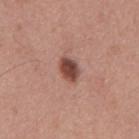workup: catalogued during a skin exam; not biopsied | image: ~15 mm tile from a whole-body skin photo | lesion diameter: ~3 mm (longest diameter) | illumination: white-light illumination | patient: male, aged approximately 55 | location: the mid back | TBP lesion metrics: a shape eccentricity near 0.75 and two-axis asymmetry of about 0.2; a lesion color around L≈46 a*≈23 b*≈25 in CIELAB, a lesion–skin lightness drop of about 15, and a normalized border contrast of about 11; an automated nevus-likeness rating near 100 out of 100 and lesion-presence confidence of about 100/100.